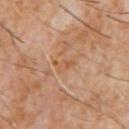notes: total-body-photography surveillance lesion; no biopsy | location: the front of the torso | image: 15 mm crop, total-body photography | lighting: cross-polarized | patient: male, in their 60s | TBP lesion metrics: an average lesion color of about L≈51 a*≈20 b*≈34 (CIELAB) and about 6 CIELAB-L* units darker than the surrounding skin | lesion size: ~2.5 mm (longest diameter).